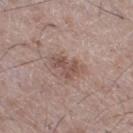biopsy status = total-body-photography surveillance lesion; no biopsy
subject = male, approximately 50 years of age
lesion size = ~3.5 mm (longest diameter)
body site = the right thigh
tile lighting = white-light illumination
automated metrics = border irregularity of about 4.5 on a 0–10 scale and a within-lesion color-variation index near 2.5/10; lesion-presence confidence of about 100/100
image = ~15 mm crop, total-body skin-cancer survey